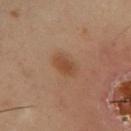Q: Was a biopsy performed?
A: total-body-photography surveillance lesion; no biopsy
Q: How large is the lesion?
A: ≈3 mm
Q: What is the imaging modality?
A: total-body-photography crop, ~15 mm field of view
Q: What lighting was used for the tile?
A: cross-polarized illumination
Q: What is the anatomic site?
A: the right upper arm
Q: What are the patient's age and sex?
A: male, aged 38 to 42